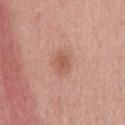Part of a total-body skin-imaging series; this lesion was reviewed on a skin check and was not flagged for biopsy. The patient is a female approximately 35 years of age. The lesion is located on the head or neck. A close-up tile cropped from a whole-body skin photograph, about 15 mm across.